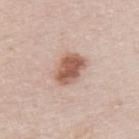Imaged during a routine full-body skin examination; the lesion was not biopsied and no histopathology is available.